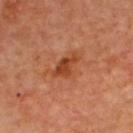This lesion was catalogued during total-body skin photography and was not selected for biopsy. A 15 mm crop from a total-body photograph taken for skin-cancer surveillance. From the upper back. A male subject aged around 60.